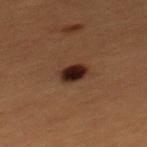follow-up: total-body-photography surveillance lesion; no biopsy
image: 15 mm crop, total-body photography
location: the mid back
patient: male, aged 48–52